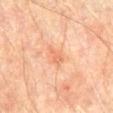Clinical impression: No biopsy was performed on this lesion — it was imaged during a full skin examination and was not determined to be concerning. Clinical summary: A region of skin cropped from a whole-body photographic capture, roughly 15 mm wide. Imaged with cross-polarized lighting. The lesion is located on the abdomen. A male subject, about 75 years old. Measured at roughly 2.5 mm in maximum diameter.